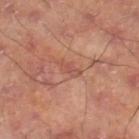Imaged during a routine full-body skin examination; the lesion was not biopsied and no histopathology is available.
The tile uses cross-polarized illumination.
A region of skin cropped from a whole-body photographic capture, roughly 15 mm wide.
The lesion-visualizer software estimated an outline eccentricity of about 0.9 (0 = round, 1 = elongated). And it measured a nevus-likeness score of about 0/100 and a detector confidence of about 80 out of 100 that the crop contains a lesion.
On the right thigh.